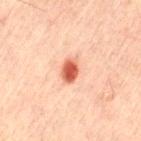{
  "biopsy_status": "not biopsied; imaged during a skin examination",
  "patient": {
    "sex": "male",
    "age_approx": 60
  },
  "lesion_size": {
    "long_diameter_mm_approx": 3.0
  },
  "image": {
    "source": "total-body photography crop",
    "field_of_view_mm": 15
  },
  "site": "lower back",
  "lighting": "cross-polarized"
}